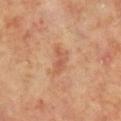The lesion was tiled from a total-body skin photograph and was not biopsied. Captured under cross-polarized illumination. The lesion is on the leg. A region of skin cropped from a whole-body photographic capture, roughly 15 mm wide. Automated image analysis of the tile measured a footprint of about 4 mm², a shape eccentricity near 0.8, and a shape-asymmetry score of about 0.5 (0 = symmetric). It also reported border irregularity of about 5 on a 0–10 scale, internal color variation of about 3 on a 0–10 scale, and radial color variation of about 1. The analysis additionally found a classifier nevus-likeness of about 0/100 and lesion-presence confidence of about 100/100. The subject is a female aged 68 to 72.